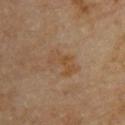The subject is a male about 85 years old or older.
A 15 mm close-up tile from a total-body photography series done for melanoma screening.
Located on the upper back.